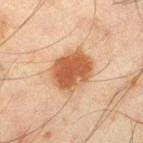notes: catalogued during a skin exam; not biopsied
imaging modality: ~15 mm tile from a whole-body skin photo
patient: male, aged around 45
tile lighting: cross-polarized
image-analysis metrics: an outline eccentricity of about 0.6 (0 = round, 1 = elongated) and a shape-asymmetry score of about 0.15 (0 = symmetric); an automated nevus-likeness rating near 100 out of 100
diameter: ~5 mm (longest diameter)
anatomic site: the left thigh